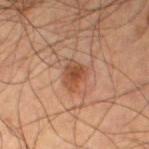acquisition=15 mm crop, total-body photography
subject=male, in their mid-50s
body site=the left thigh